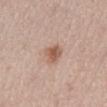image-analysis metrics=a lesion color around L≈56 a*≈20 b*≈29 in CIELAB, about 11 CIELAB-L* units darker than the surrounding skin, and a normalized border contrast of about 8; a nevus-likeness score of about 95/100
acquisition=15 mm crop, total-body photography
body site=the abdomen
patient=female, roughly 65 years of age